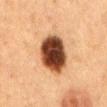Assessment:
Imaged during a routine full-body skin examination; the lesion was not biopsied and no histopathology is available.
Image and clinical context:
Located on the chest. A close-up tile cropped from a whole-body skin photograph, about 15 mm across. Automated image analysis of the tile measured a lesion area of about 18 mm², an eccentricity of roughly 0.7, and two-axis asymmetry of about 0.1. And it measured a lesion color around L≈36 a*≈21 b*≈29 in CIELAB, roughly 23 lightness units darker than nearby skin, and a normalized border contrast of about 17.5. And it measured a nevus-likeness score of about 95/100 and a detector confidence of about 100 out of 100 that the crop contains a lesion. The recorded lesion diameter is about 5.5 mm. A female patient aged 58 to 62. The tile uses cross-polarized illumination.